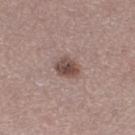biopsy status=imaged on a skin check; not biopsied
automated lesion analysis=a symmetry-axis asymmetry near 0.25; an average lesion color of about L≈47 a*≈17 b*≈21 (CIELAB), roughly 13 lightness units darker than nearby skin, and a normalized border contrast of about 9
tile lighting=white-light
body site=the leg
size=≈2.5 mm
acquisition=total-body-photography crop, ~15 mm field of view
patient=female, approximately 25 years of age